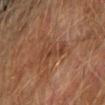Part of a total-body skin-imaging series; this lesion was reviewed on a skin check and was not flagged for biopsy. The lesion is on the right upper arm. A male patient, about 75 years old. A lesion tile, about 15 mm wide, cut from a 3D total-body photograph.